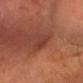Case summary:
• notes — no biopsy performed (imaged during a skin exam)
• patient — male, in their mid- to late 60s
• lesion diameter — about 3 mm
• lighting — cross-polarized
• location — the head or neck
• imaging modality — ~15 mm tile from a whole-body skin photo